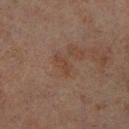The lesion was tiled from a total-body skin photograph and was not biopsied.
The patient is a male about 70 years old.
A 15 mm close-up extracted from a 3D total-body photography capture.
The lesion is located on the right lower leg.
Longest diameter approximately 3 mm.